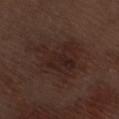Impression:
The lesion was photographed on a routine skin check and not biopsied; there is no pathology result.
Background:
Longest diameter approximately 7 mm. The lesion is on the right thigh. A male patient aged 68 to 72. Captured under white-light illumination. Cropped from a total-body skin-imaging series; the visible field is about 15 mm. Automated image analysis of the tile measured a shape eccentricity near 0.65 and two-axis asymmetry of about 0.4. And it measured border irregularity of about 6 on a 0–10 scale and a color-variation rating of about 3/10. It also reported a nevus-likeness score of about 0/100 and lesion-presence confidence of about 100/100.